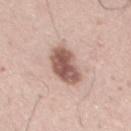No biopsy was performed on this lesion — it was imaged during a full skin examination and was not determined to be concerning.
The subject is a male aged around 60.
On the chest.
A region of skin cropped from a whole-body photographic capture, roughly 15 mm wide.
Captured under white-light illumination.
The lesion's longest dimension is about 4.5 mm.